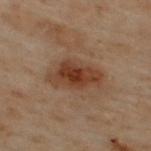On the upper back.
A male patient roughly 50 years of age.
A lesion tile, about 15 mm wide, cut from a 3D total-body photograph.
The lesion's longest dimension is about 5.5 mm.